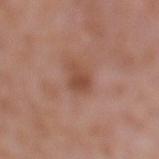Imaged during a routine full-body skin examination; the lesion was not biopsied and no histopathology is available.
The total-body-photography lesion software estimated an average lesion color of about L≈48 a*≈23 b*≈30 (CIELAB), roughly 8 lightness units darker than nearby skin, and a normalized lesion–skin contrast near 7. The software also gave a border-irregularity index near 3/10, internal color variation of about 3 on a 0–10 scale, and a peripheral color-asymmetry measure near 1.
A roughly 15 mm field-of-view crop from a total-body skin photograph.
Captured under white-light illumination.
The lesion is located on the left lower leg.
The lesion's longest dimension is about 2.5 mm.
A female subject, aged 38–42.